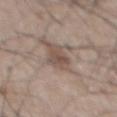  biopsy_status: not biopsied; imaged during a skin examination
  patient:
    sex: male
    age_approx: 55
  lesion_size:
    long_diameter_mm_approx: 3.0
  site: front of the torso
  image:
    source: total-body photography crop
    field_of_view_mm: 15
  automated_metrics:
    area_mm2_approx: 3.5
    eccentricity: 0.8
    shape_asymmetry: 0.45
    cielab_L: 49
    cielab_a: 15
    cielab_b: 23
    vs_skin_darker_L: 9.0
    vs_skin_contrast_norm: 6.5
    color_variation_0_10: 2.0